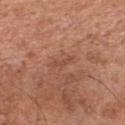Image and clinical context: Cropped from a whole-body photographic skin survey; the tile spans about 15 mm. A male patient approximately 65 years of age. Longest diameter approximately 2.5 mm. Located on the chest. This is a white-light tile. The total-body-photography lesion software estimated a footprint of about 2 mm², an outline eccentricity of about 0.95 (0 = round, 1 = elongated), and a symmetry-axis asymmetry near 0.4. The analysis additionally found a mean CIELAB color near L≈48 a*≈24 b*≈30, a lesion–skin lightness drop of about 6, and a normalized border contrast of about 4.5. The software also gave a border-irregularity index near 5/10, a within-lesion color-variation index near 0/10, and peripheral color asymmetry of about 0. The analysis additionally found an automated nevus-likeness rating near 0 out of 100 and a lesion-detection confidence of about 100/100.